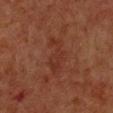{
  "biopsy_status": "not biopsied; imaged during a skin examination",
  "lighting": "cross-polarized",
  "site": "front of the torso",
  "image": {
    "source": "total-body photography crop",
    "field_of_view_mm": 15
  },
  "automated_metrics": {
    "cielab_L": 27,
    "cielab_a": 21,
    "cielab_b": 25,
    "vs_skin_darker_L": 4.0,
    "vs_skin_contrast_norm": 5.0,
    "border_irregularity_0_10": 6.0,
    "color_variation_0_10": 1.5,
    "peripheral_color_asymmetry": 0.5,
    "lesion_detection_confidence_0_100": 100
  },
  "patient": {
    "sex": "female",
    "age_approx": 55
  },
  "lesion_size": {
    "long_diameter_mm_approx": 5.0
  }
}